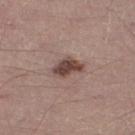Clinical impression: Captured during whole-body skin photography for melanoma surveillance; the lesion was not biopsied. Clinical summary: Approximately 3.5 mm at its widest. Captured under white-light illumination. The lesion is located on the leg. The lesion-visualizer software estimated an area of roughly 6 mm², an outline eccentricity of about 0.8 (0 = round, 1 = elongated), and a symmetry-axis asymmetry near 0.35. A close-up tile cropped from a whole-body skin photograph, about 15 mm across. A male patient, roughly 40 years of age.